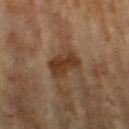Findings:
– workup — catalogued during a skin exam; not biopsied
– location — the left forearm
– image source — ~15 mm crop, total-body skin-cancer survey
– subject — female, approximately 80 years of age
– lighting — cross-polarized illumination